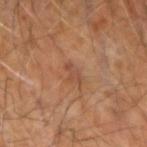| feature | finding |
|---|---|
| workup | imaged on a skin check; not biopsied |
| automated metrics | an eccentricity of roughly 0.9; about 7 CIELAB-L* units darker than the surrounding skin and a normalized border contrast of about 5.5 |
| anatomic site | the arm |
| subject | male, aged approximately 60 |
| image | ~15 mm tile from a whole-body skin photo |